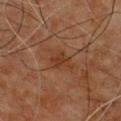| feature | finding |
|---|---|
| biopsy status | no biopsy performed (imaged during a skin exam) |
| lighting | cross-polarized illumination |
| lesion size | about 3 mm |
| imaging modality | total-body-photography crop, ~15 mm field of view |
| anatomic site | the front of the torso |
| subject | male, aged 58 to 62 |